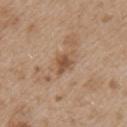No biopsy was performed on this lesion — it was imaged during a full skin examination and was not determined to be concerning. Cropped from a total-body skin-imaging series; the visible field is about 15 mm. Located on the right upper arm. The lesion's longest dimension is about 2.5 mm. The lesion-visualizer software estimated a lesion area of about 4.5 mm², an eccentricity of roughly 0.65, and a shape-asymmetry score of about 0.2 (0 = symmetric). Imaged with white-light lighting. The subject is a male aged around 50.